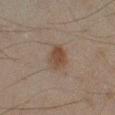| field | value |
|---|---|
| notes | total-body-photography surveillance lesion; no biopsy |
| diameter | ~3 mm (longest diameter) |
| tile lighting | cross-polarized illumination |
| patient | male, aged around 45 |
| imaging modality | ~15 mm tile from a whole-body skin photo |
| site | the left lower leg |